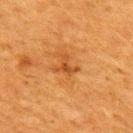This lesion was catalogued during total-body skin photography and was not selected for biopsy. The patient is a female in their 40s. This image is a 15 mm lesion crop taken from a total-body photograph. Located on the upper back.